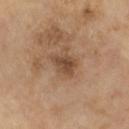Clinical impression: Recorded during total-body skin imaging; not selected for excision or biopsy. Acquisition and patient details: A male subject, aged around 65. Cropped from a whole-body photographic skin survey; the tile spans about 15 mm. Imaged with cross-polarized lighting. The recorded lesion diameter is about 3.5 mm.